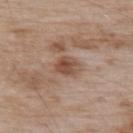{"biopsy_status": "not biopsied; imaged during a skin examination", "lesion_size": {"long_diameter_mm_approx": 2.5}, "site": "upper back", "image": {"source": "total-body photography crop", "field_of_view_mm": 15}, "patient": {"sex": "male", "age_approx": 55}, "lighting": "white-light"}